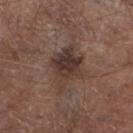No biopsy was performed on this lesion — it was imaged during a full skin examination and was not determined to be concerning. The patient is a male in their mid- to late 50s. Cropped from a total-body skin-imaging series; the visible field is about 15 mm. Captured under white-light illumination. Located on the left lower leg. The recorded lesion diameter is about 4.5 mm.